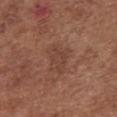Assessment:
The lesion was photographed on a routine skin check and not biopsied; there is no pathology result.
Context:
Longest diameter approximately 4 mm. A female patient, in their mid-70s. Located on the chest. Imaged with white-light lighting. Automated tile analysis of the lesion measured a lesion area of about 8 mm², an outline eccentricity of about 0.7 (0 = round, 1 = elongated), and a symmetry-axis asymmetry near 0.45. The software also gave a border-irregularity rating of about 4.5/10, a within-lesion color-variation index near 1.5/10, and peripheral color asymmetry of about 0.5. This image is a 15 mm lesion crop taken from a total-body photograph.